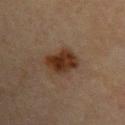Part of a total-body skin-imaging series; this lesion was reviewed on a skin check and was not flagged for biopsy. The lesion-visualizer software estimated a lesion area of about 9 mm², an outline eccentricity of about 0.8 (0 = round, 1 = elongated), and a symmetry-axis asymmetry near 0.25. And it measured a lesion color around L≈27 a*≈16 b*≈25 in CIELAB, a lesion–skin lightness drop of about 11, and a normalized lesion–skin contrast near 11.5. The software also gave a color-variation rating of about 3.5/10 and a peripheral color-asymmetry measure near 1. The lesion is on the upper back. The patient is a male aged 43–47. Measured at roughly 4.5 mm in maximum diameter. A lesion tile, about 15 mm wide, cut from a 3D total-body photograph. Captured under cross-polarized illumination.